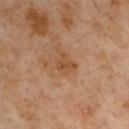Recorded during total-body skin imaging; not selected for excision or biopsy. The total-body-photography lesion software estimated lesion-presence confidence of about 100/100. The recorded lesion diameter is about 3 mm. A male subject, aged approximately 55. A region of skin cropped from a whole-body photographic capture, roughly 15 mm wide. On the left upper arm. Imaged with cross-polarized lighting.